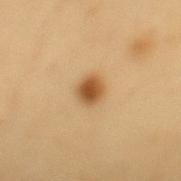Clinical impression: Recorded during total-body skin imaging; not selected for excision or biopsy. Background: The recorded lesion diameter is about 2.5 mm. Automated image analysis of the tile measured a classifier nevus-likeness of about 100/100. A male subject in their mid- to late 50s. On the back. A lesion tile, about 15 mm wide, cut from a 3D total-body photograph. This is a cross-polarized tile.